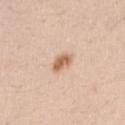Q: Was a biopsy performed?
A: total-body-photography surveillance lesion; no biopsy